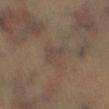biopsy_status: not biopsied; imaged during a skin examination
patient:
  sex: male
  age_approx: 45
lesion_size:
  long_diameter_mm_approx: 3.0
site: right lower leg
automated_metrics:
  cielab_L: 39
  cielab_a: 12
  cielab_b: 20
  vs_skin_darker_L: 4.0
  vs_skin_contrast_norm: 4.5
  border_irregularity_0_10: 2.5
  color_variation_0_10: 3.0
  peripheral_color_asymmetry: 1.0
image:
  source: total-body photography crop
  field_of_view_mm: 15